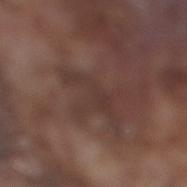Assessment:
Part of a total-body skin-imaging series; this lesion was reviewed on a skin check and was not flagged for biopsy.
Clinical summary:
Automated tile analysis of the lesion measured a lesion area of about 5 mm², an outline eccentricity of about 0.95 (0 = round, 1 = elongated), and a symmetry-axis asymmetry near 0.6. The software also gave an average lesion color of about L≈34 a*≈17 b*≈20 (CIELAB), a lesion–skin lightness drop of about 5, and a normalized border contrast of about 5.5. The patient is a male in their mid- to late 70s. Located on the leg. Imaged with white-light lighting. A close-up tile cropped from a whole-body skin photograph, about 15 mm across.